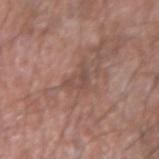| feature | finding |
|---|---|
| workup | total-body-photography surveillance lesion; no biopsy |
| body site | the left forearm |
| patient | male, approximately 55 years of age |
| image source | ~15 mm crop, total-body skin-cancer survey |